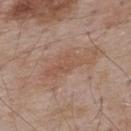A male subject aged approximately 55. Cropped from a total-body skin-imaging series; the visible field is about 15 mm. Longest diameter approximately 5.5 mm. Located on the upper back.